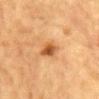Q: Was this lesion biopsied?
A: imaged on a skin check; not biopsied
Q: What is the anatomic site?
A: the chest
Q: What did automated image analysis measure?
A: an eccentricity of roughly 0.75 and a shape-asymmetry score of about 0.25 (0 = symmetric); a detector confidence of about 100 out of 100 that the crop contains a lesion
Q: Illumination type?
A: cross-polarized
Q: Who is the patient?
A: male, in their mid- to late 80s or older
Q: How was this image acquired?
A: ~15 mm tile from a whole-body skin photo
Q: How large is the lesion?
A: ≈3 mm